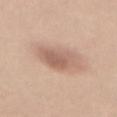No biopsy was performed on this lesion — it was imaged during a full skin examination and was not determined to be concerning.
A close-up tile cropped from a whole-body skin photograph, about 15 mm across.
Longest diameter approximately 5.5 mm.
From the mid back.
A female patient, aged approximately 35.